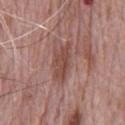Clinical impression: The lesion was photographed on a routine skin check and not biopsied; there is no pathology result. Image and clinical context: The lesion is located on the chest. The tile uses white-light illumination. A male patient, aged 68 to 72. Cropped from a total-body skin-imaging series; the visible field is about 15 mm.